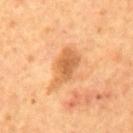This lesion was catalogued during total-body skin photography and was not selected for biopsy.
A 15 mm close-up tile from a total-body photography series done for melanoma screening.
The subject is a male aged 53 to 57.
From the mid back.
Imaged with cross-polarized lighting.
Longest diameter approximately 5 mm.